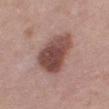Findings:
* anatomic site — the leg
* tile lighting — white-light illumination
* image source — total-body-photography crop, ~15 mm field of view
* patient — female, aged around 40
* size — ≈6 mm